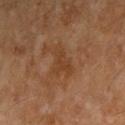workup: catalogued during a skin exam; not biopsied
subject: male, aged around 55
image source: ~15 mm crop, total-body skin-cancer survey
site: the arm
lighting: cross-polarized
automated lesion analysis: a lesion color around L≈37 a*≈20 b*≈32 in CIELAB; a detector confidence of about 100 out of 100 that the crop contains a lesion